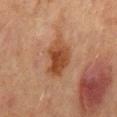workup=no biopsy performed (imaged during a skin exam); acquisition=~15 mm tile from a whole-body skin photo; tile lighting=cross-polarized illumination; lesion size=≈5.5 mm; location=the abdomen; subject=male, approximately 65 years of age.